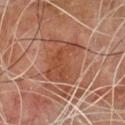  biopsy_status: not biopsied; imaged during a skin examination
  patient:
    sex: male
    age_approx: 65
  lighting: cross-polarized
  site: front of the torso
  lesion_size:
    long_diameter_mm_approx: 5.5
  automated_metrics:
    cielab_L: 48
    cielab_a: 26
    cielab_b: 33
    vs_skin_darker_L: 8.0
    vs_skin_contrast_norm: 7.0
    color_variation_0_10: 3.0
    peripheral_color_asymmetry: 1.0
    nevus_likeness_0_100: 10
    lesion_detection_confidence_0_100: 90
  image:
    source: total-body photography crop
    field_of_view_mm: 15